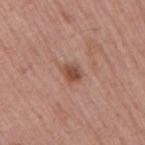Clinical impression: This lesion was catalogued during total-body skin photography and was not selected for biopsy. Acquisition and patient details: About 2.5 mm across. Cropped from a total-body skin-imaging series; the visible field is about 15 mm. Imaged with white-light lighting. The lesion is on the right upper arm. A male patient aged approximately 55. The lesion-visualizer software estimated a mean CIELAB color near L≈49 a*≈23 b*≈28 and roughly 11 lightness units darker than nearby skin. And it measured a border-irregularity rating of about 1.5/10, internal color variation of about 3 on a 0–10 scale, and peripheral color asymmetry of about 1. The software also gave a classifier nevus-likeness of about 90/100 and a lesion-detection confidence of about 100/100.